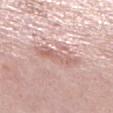Notes:
– site · the leg
– acquisition · ~15 mm tile from a whole-body skin photo
– subject · female, about 40 years old